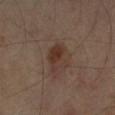Case summary:
* follow-up — catalogued during a skin exam; not biopsied
* tile lighting — cross-polarized
* image-analysis metrics — an eccentricity of roughly 0.75 and two-axis asymmetry of about 0.3
* anatomic site — the right forearm
* subject — roughly 55 years of age
* image — ~15 mm crop, total-body skin-cancer survey
* diameter — ~4 mm (longest diameter)